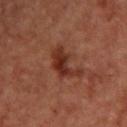Assessment:
Captured during whole-body skin photography for melanoma surveillance; the lesion was not biopsied.
Context:
On the upper back. A 15 mm close-up tile from a total-body photography series done for melanoma screening. A female subject, in their mid-40s. Captured under cross-polarized illumination. The recorded lesion diameter is about 4.5 mm. An algorithmic analysis of the crop reported a footprint of about 8 mm², an outline eccentricity of about 0.8 (0 = round, 1 = elongated), and two-axis asymmetry of about 0.5. And it measured a border-irregularity rating of about 6.5/10. The software also gave a nevus-likeness score of about 20/100.